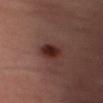follow-up: total-body-photography surveillance lesion; no biopsy | TBP lesion metrics: a lesion area of about 6 mm², a shape eccentricity near 0.7, and a shape-asymmetry score of about 0.15 (0 = symmetric); a mean CIELAB color near L≈28 a*≈22 b*≈22, roughly 12 lightness units darker than nearby skin, and a normalized lesion–skin contrast near 11.5; a border-irregularity rating of about 1.5/10 and radial color variation of about 1.5 | patient: female, about 55 years old | body site: the abdomen | tile lighting: cross-polarized | image: total-body-photography crop, ~15 mm field of view | lesion diameter: ~3 mm (longest diameter).